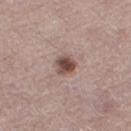biopsy status: imaged on a skin check; not biopsied | image source: total-body-photography crop, ~15 mm field of view | subject: female, aged 48–52 | size: ~3 mm (longest diameter) | location: the right thigh.